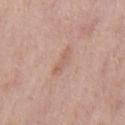Clinical impression: Part of a total-body skin-imaging series; this lesion was reviewed on a skin check and was not flagged for biopsy. Image and clinical context: On the mid back. A lesion tile, about 15 mm wide, cut from a 3D total-body photograph. Captured under white-light illumination. The subject is a male aged 73–77. Approximately 3.5 mm at its widest.